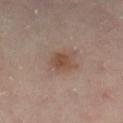  biopsy_status: not biopsied; imaged during a skin examination
  patient:
    sex: female
    age_approx: 55
  lighting: cross-polarized
  image:
    source: total-body photography crop
    field_of_view_mm: 15
  site: right lower leg